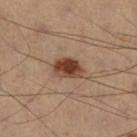{"image": {"source": "total-body photography crop", "field_of_view_mm": 15}, "patient": {"sex": "male", "age_approx": 50}, "site": "left leg", "lesion_size": {"long_diameter_mm_approx": 3.5}, "automated_metrics": {"cielab_L": 40, "cielab_a": 20, "cielab_b": 28, "vs_skin_darker_L": 15.0, "vs_skin_contrast_norm": 11.5, "nevus_likeness_0_100": 100, "lesion_detection_confidence_0_100": 100}, "lighting": "cross-polarized"}